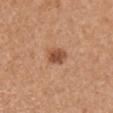Approximately 2.5 mm at its widest.
The tile uses white-light illumination.
The lesion is on the right upper arm.
Automated image analysis of the tile measured a footprint of about 4.5 mm², a shape eccentricity near 0.65, and two-axis asymmetry of about 0.25. The software also gave an average lesion color of about L≈50 a*≈23 b*≈33 (CIELAB) and a lesion-to-skin contrast of about 9 (normalized; higher = more distinct). The software also gave a border-irregularity rating of about 2/10, a within-lesion color-variation index near 4/10, and radial color variation of about 1.5.
A female subject, in their mid- to late 40s.
A lesion tile, about 15 mm wide, cut from a 3D total-body photograph.